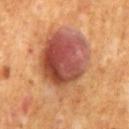Q: Was a biopsy performed?
A: catalogued during a skin exam; not biopsied
Q: How large is the lesion?
A: ~10 mm (longest diameter)
Q: What kind of image is this?
A: ~15 mm crop, total-body skin-cancer survey
Q: Where on the body is the lesion?
A: the mid back
Q: How was the tile lit?
A: cross-polarized illumination
Q: Who is the patient?
A: male, aged 68 to 72